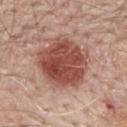TBP lesion metrics: a footprint of about 27 mm², an eccentricity of roughly 0.4, and a shape-asymmetry score of about 0.15 (0 = symmetric); border irregularity of about 1.5 on a 0–10 scale, a within-lesion color-variation index near 5.5/10, and peripheral color asymmetry of about 2 | location: the back | imaging modality: ~15 mm tile from a whole-body skin photo | patient: male, in their 70s | lighting: white-light | size: about 6 mm.